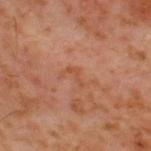| feature | finding |
|---|---|
| notes | no biopsy performed (imaged during a skin exam) |
| patient | male, approximately 60 years of age |
| site | the upper back |
| TBP lesion metrics | a lesion area of about 2 mm², an outline eccentricity of about 0.9 (0 = round, 1 = elongated), and two-axis asymmetry of about 0.6; a mean CIELAB color near L≈47 a*≈24 b*≈33, a lesion–skin lightness drop of about 5, and a normalized border contrast of about 5.5; border irregularity of about 6.5 on a 0–10 scale, a color-variation rating of about 0/10, and a peripheral color-asymmetry measure near 0; an automated nevus-likeness rating near 0 out of 100 and a detector confidence of about 100 out of 100 that the crop contains a lesion |
| image | ~15 mm tile from a whole-body skin photo |
| size | about 2.5 mm |